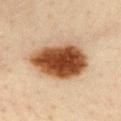{"biopsy_status": "not biopsied; imaged during a skin examination", "lighting": "cross-polarized", "patient": {"sex": "female", "age_approx": 45}, "lesion_size": {"long_diameter_mm_approx": 7.5}, "automated_metrics": {"cielab_L": 43, "cielab_a": 20, "cielab_b": 32, "vs_skin_darker_L": 21.0}, "site": "chest", "image": {"source": "total-body photography crop", "field_of_view_mm": 15}}